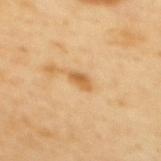{
  "biopsy_status": "not biopsied; imaged during a skin examination",
  "image": {
    "source": "total-body photography crop",
    "field_of_view_mm": 15
  },
  "patient": {
    "sex": "female",
    "age_approx": 55
  },
  "site": "mid back"
}